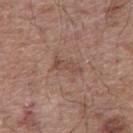{"biopsy_status": "not biopsied; imaged during a skin examination", "lighting": "white-light", "site": "mid back", "automated_metrics": {"border_irregularity_0_10": 6.0, "color_variation_0_10": 0.0, "peripheral_color_asymmetry": 0.0, "nevus_likeness_0_100": 0, "lesion_detection_confidence_0_100": 100}, "patient": {"sex": "male", "age_approx": 55}, "image": {"source": "total-body photography crop", "field_of_view_mm": 15}, "lesion_size": {"long_diameter_mm_approx": 3.5}}